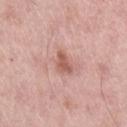Q: Was this lesion biopsied?
A: catalogued during a skin exam; not biopsied